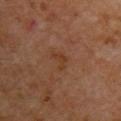A male subject about 60 years old. An algorithmic analysis of the crop reported a mean CIELAB color near L≈33 a*≈19 b*≈28, roughly 5 lightness units darker than nearby skin, and a lesion-to-skin contrast of about 5.5 (normalized; higher = more distinct). It also reported a border-irregularity rating of about 5.5/10, a within-lesion color-variation index near 1/10, and a peripheral color-asymmetry measure near 0.5. A lesion tile, about 15 mm wide, cut from a 3D total-body photograph. Captured under cross-polarized illumination. From the back.